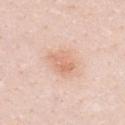Captured during whole-body skin photography for melanoma surveillance; the lesion was not biopsied.
Automated image analysis of the tile measured border irregularity of about 3 on a 0–10 scale, internal color variation of about 3 on a 0–10 scale, and radial color variation of about 1. It also reported a nevus-likeness score of about 55/100 and a lesion-detection confidence of about 100/100.
A close-up tile cropped from a whole-body skin photograph, about 15 mm across.
A female patient, approximately 30 years of age.
From the chest.
Imaged with white-light lighting.